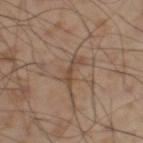Imaged during a routine full-body skin examination; the lesion was not biopsied and no histopathology is available. Measured at roughly 4 mm in maximum diameter. Imaged with cross-polarized lighting. A 15 mm crop from a total-body photograph taken for skin-cancer surveillance. A male patient about 55 years old. The lesion is on the leg.